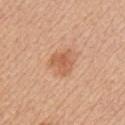follow-up: imaged on a skin check; not biopsied | diameter: ≈3 mm | lighting: white-light | automated lesion analysis: an average lesion color of about L≈59 a*≈25 b*≈35 (CIELAB), a lesion–skin lightness drop of about 9, and a lesion-to-skin contrast of about 6.5 (normalized; higher = more distinct); a border-irregularity index near 3.5/10, a color-variation rating of about 3/10, and radial color variation of about 1; a classifier nevus-likeness of about 55/100 and a detector confidence of about 100 out of 100 that the crop contains a lesion | image: total-body-photography crop, ~15 mm field of view | location: the chest | patient: female, in their 40s.